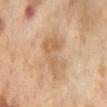Q: Was this lesion biopsied?
A: imaged on a skin check; not biopsied
Q: Illumination type?
A: cross-polarized illumination
Q: Lesion size?
A: about 5 mm
Q: Where on the body is the lesion?
A: the abdomen
Q: What is the imaging modality?
A: ~15 mm tile from a whole-body skin photo
Q: Who is the patient?
A: female, roughly 55 years of age
Q: What did automated image analysis measure?
A: a mean CIELAB color near L≈63 a*≈19 b*≈35, roughly 8 lightness units darker than nearby skin, and a normalized border contrast of about 5.5; a nevus-likeness score of about 0/100 and a lesion-detection confidence of about 100/100